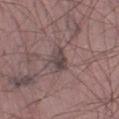Context:
On the left lower leg. The total-body-photography lesion software estimated a lesion area of about 4.5 mm² and two-axis asymmetry of about 0.25. The software also gave a border-irregularity index near 2/10 and a within-lesion color-variation index near 3.5/10. And it measured a classifier nevus-likeness of about 0/100 and a lesion-detection confidence of about 70/100. Measured at roughly 2.5 mm in maximum diameter. A male patient, aged 48–52. A close-up tile cropped from a whole-body skin photograph, about 15 mm across.